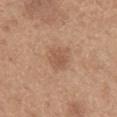No biopsy was performed on this lesion — it was imaged during a full skin examination and was not determined to be concerning. A male patient, aged approximately 65. A 15 mm crop from a total-body photograph taken for skin-cancer surveillance. The lesion's longest dimension is about 3 mm. The lesion is located on the mid back.